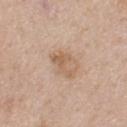Recorded during total-body skin imaging; not selected for excision or biopsy.